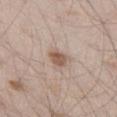{
  "biopsy_status": "not biopsied; imaged during a skin examination",
  "patient": {
    "sex": "male",
    "age_approx": 45
  },
  "lesion_size": {
    "long_diameter_mm_approx": 2.5
  },
  "lighting": "white-light",
  "site": "left thigh",
  "image": {
    "source": "total-body photography crop",
    "field_of_view_mm": 15
  }
}